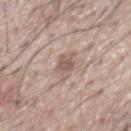workup: imaged on a skin check; not biopsied.